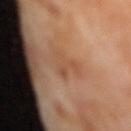Assessment:
The lesion was tiled from a total-body skin photograph and was not biopsied.
Clinical summary:
A female subject in their mid- to late 50s. The tile uses cross-polarized illumination. Automated tile analysis of the lesion measured a shape eccentricity near 0.6 and a symmetry-axis asymmetry near 0.75. The software also gave an average lesion color of about L≈52 a*≈22 b*≈34 (CIELAB) and a lesion–skin lightness drop of about 6. The analysis additionally found an automated nevus-likeness rating near 0 out of 100. The lesion is located on the mid back. Cropped from a total-body skin-imaging series; the visible field is about 15 mm. The lesion's longest dimension is about 3.5 mm.